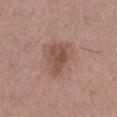Part of a total-body skin-imaging series; this lesion was reviewed on a skin check and was not flagged for biopsy.
A female subject, in their mid- to late 50s.
The lesion is on the right lower leg.
A 15 mm close-up extracted from a 3D total-body photography capture.